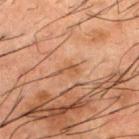Q: What kind of image is this?
A: total-body-photography crop, ~15 mm field of view
Q: What is the anatomic site?
A: the upper back
Q: What lighting was used for the tile?
A: cross-polarized
Q: Who is the patient?
A: male, aged 48 to 52